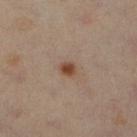Case summary:
• biopsy status: catalogued during a skin exam; not biopsied
• location: the right lower leg
• image: 15 mm crop, total-body photography
• patient: female, aged around 40
• diameter: ~2 mm (longest diameter)